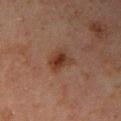follow-up: no biopsy performed (imaged during a skin exam)
imaging modality: 15 mm crop, total-body photography
patient: male, approximately 45 years of age
anatomic site: the right upper arm